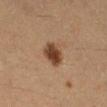Acquisition and patient details:
The lesion-visualizer software estimated a lesion color around L≈39 a*≈18 b*≈29 in CIELAB, a lesion–skin lightness drop of about 13, and a normalized lesion–skin contrast near 10.5. Longest diameter approximately 3 mm. On the right lower leg. A roughly 15 mm field-of-view crop from a total-body skin photograph. Imaged with cross-polarized lighting. The patient is a female approximately 30 years of age.